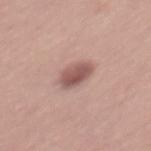No biopsy was performed on this lesion — it was imaged during a full skin examination and was not determined to be concerning.
The lesion is located on the mid back.
An algorithmic analysis of the crop reported a lesion area of about 7.5 mm² and an eccentricity of roughly 0.75.
The tile uses white-light illumination.
Approximately 3.5 mm at its widest.
The subject is a female aged 48–52.
A close-up tile cropped from a whole-body skin photograph, about 15 mm across.